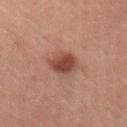Captured during whole-body skin photography for melanoma surveillance; the lesion was not biopsied.
A 15 mm crop from a total-body photograph taken for skin-cancer surveillance.
A female patient roughly 50 years of age.
The lesion is located on the lower back.
An algorithmic analysis of the crop reported a footprint of about 7 mm², an outline eccentricity of about 0.55 (0 = round, 1 = elongated), and a symmetry-axis asymmetry near 0.2. The analysis additionally found a border-irregularity rating of about 1.5/10, internal color variation of about 2.5 on a 0–10 scale, and radial color variation of about 0.5. And it measured an automated nevus-likeness rating near 95 out of 100.
About 3 mm across.